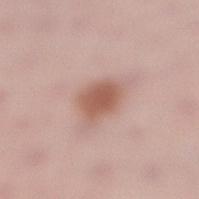Assessment: Part of a total-body skin-imaging series; this lesion was reviewed on a skin check and was not flagged for biopsy. Image and clinical context: Captured under white-light illumination. The subject is a female aged approximately 30. On the right lower leg. A 15 mm close-up extracted from a 3D total-body photography capture.